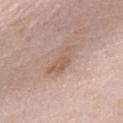An algorithmic analysis of the crop reported a border-irregularity index near 4.5/10, a color-variation rating of about 3/10, and a peripheral color-asymmetry measure near 1. It also reported a classifier nevus-likeness of about 0/100 and a lesion-detection confidence of about 100/100. A female patient, about 65 years old. Imaged with white-light lighting. Located on the chest. The lesion's longest dimension is about 4.5 mm. Cropped from a whole-body photographic skin survey; the tile spans about 15 mm.